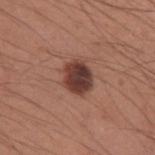notes: catalogued during a skin exam; not biopsied | size: ~4 mm (longest diameter) | image-analysis metrics: a footprint of about 9.5 mm², a shape eccentricity near 0.4, and two-axis asymmetry of about 0.2; border irregularity of about 2 on a 0–10 scale, a within-lesion color-variation index near 5/10, and peripheral color asymmetry of about 1.5 | subject: male, approximately 35 years of age | acquisition: 15 mm crop, total-body photography | anatomic site: the left upper arm.